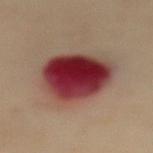– follow-up — imaged on a skin check; not biopsied